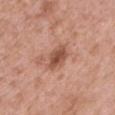Assessment:
The lesion was tiled from a total-body skin photograph and was not biopsied.
Acquisition and patient details:
A close-up tile cropped from a whole-body skin photograph, about 15 mm across. On the left upper arm. The recorded lesion diameter is about 3 mm. A male subject, aged 48–52. This is a white-light tile.